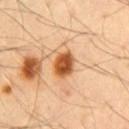The subject is a male about 55 years old.
The lesion's longest dimension is about 3.5 mm.
The lesion is on the chest.
A 15 mm close-up extracted from a 3D total-body photography capture.